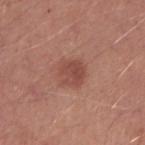No biopsy was performed on this lesion — it was imaged during a full skin examination and was not determined to be concerning.
The lesion is located on the right upper arm.
A 15 mm crop from a total-body photograph taken for skin-cancer surveillance.
This is a white-light tile.
Automated image analysis of the tile measured an eccentricity of roughly 0.45 and a shape-asymmetry score of about 0.25 (0 = symmetric). The analysis additionally found a lesion–skin lightness drop of about 9 and a normalized lesion–skin contrast near 6.5.
The lesion's longest dimension is about 3 mm.
A male patient roughly 25 years of age.